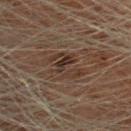{"biopsy_status": "not biopsied; imaged during a skin examination", "automated_metrics": {"color_variation_0_10": 5.0, "nevus_likeness_0_100": 0, "lesion_detection_confidence_0_100": 70}, "image": {"source": "total-body photography crop", "field_of_view_mm": 15}, "lighting": "cross-polarized", "site": "head or neck", "patient": {"sex": "male", "age_approx": 70}, "lesion_size": {"long_diameter_mm_approx": 5.5}}